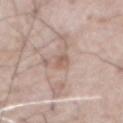Imaged during a routine full-body skin examination; the lesion was not biopsied and no histopathology is available. The lesion's longest dimension is about 2.5 mm. Imaged with white-light lighting. A male subject approximately 80 years of age. On the abdomen. Cropped from a whole-body photographic skin survey; the tile spans about 15 mm.